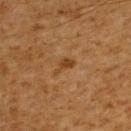Case summary:
• follow-up — catalogued during a skin exam; not biopsied
• lesion size — about 2.5 mm
• subject — male, roughly 60 years of age
• anatomic site — the upper back
• image source — total-body-photography crop, ~15 mm field of view